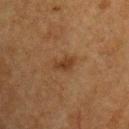Case summary:
– biopsy status: imaged on a skin check; not biopsied
– anatomic site: the chest
– size: about 2.5 mm
– subject: male, aged 73–77
– image: ~15 mm tile from a whole-body skin photo